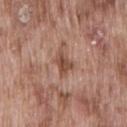Case summary:
– workup: catalogued during a skin exam; not biopsied
– subject: male, roughly 75 years of age
– automated lesion analysis: a footprint of about 5 mm², an outline eccentricity of about 0.75 (0 = round, 1 = elongated), and a symmetry-axis asymmetry near 0.35; an average lesion color of about L≈49 a*≈21 b*≈28 (CIELAB), a lesion–skin lightness drop of about 10, and a normalized border contrast of about 7.5; border irregularity of about 3.5 on a 0–10 scale, a within-lesion color-variation index near 3/10, and peripheral color asymmetry of about 1; a nevus-likeness score of about 0/100
– imaging modality: 15 mm crop, total-body photography
– site: the back
– tile lighting: white-light illumination